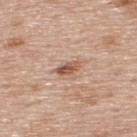biopsy status — imaged on a skin check; not biopsied
anatomic site — the upper back
illumination — white-light
lesion size — ≈2.5 mm
automated lesion analysis — a lesion area of about 3.5 mm², an eccentricity of roughly 0.8, and two-axis asymmetry of about 0.25; a normalized border contrast of about 8.5
image source — ~15 mm crop, total-body skin-cancer survey
subject — male, in their mid-70s